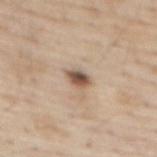biopsy status: imaged on a skin check; not biopsied
lighting: white-light
subject: male, aged 68–72
anatomic site: the abdomen
TBP lesion metrics: an area of roughly 3.5 mm², an eccentricity of roughly 0.7, and a shape-asymmetry score of about 0.3 (0 = symmetric); a lesion color around L≈53 a*≈17 b*≈27 in CIELAB, roughly 16 lightness units darker than nearby skin, and a lesion-to-skin contrast of about 11 (normalized; higher = more distinct); a border-irregularity rating of about 2.5/10, a within-lesion color-variation index near 4.5/10, and peripheral color asymmetry of about 1.5
image source: ~15 mm tile from a whole-body skin photo